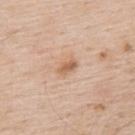This lesion was catalogued during total-body skin photography and was not selected for biopsy.
This image is a 15 mm lesion crop taken from a total-body photograph.
The recorded lesion diameter is about 2.5 mm.
A male patient aged approximately 60.
The tile uses white-light illumination.
The total-body-photography lesion software estimated a mean CIELAB color near L≈62 a*≈20 b*≈33 and about 10 CIELAB-L* units darker than the surrounding skin. It also reported an automated nevus-likeness rating near 45 out of 100.
On the upper back.